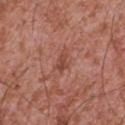Part of a total-body skin-imaging series; this lesion was reviewed on a skin check and was not flagged for biopsy.
Cropped from a whole-body photographic skin survey; the tile spans about 15 mm.
The lesion is on the chest.
A male subject, in their mid-40s.